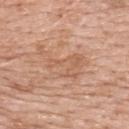biopsy status: catalogued during a skin exam; not biopsied | illumination: white-light illumination | image: 15 mm crop, total-body photography | TBP lesion metrics: a shape eccentricity near 0.85 and a symmetry-axis asymmetry near 0.45; an average lesion color of about L≈60 a*≈21 b*≈32 (CIELAB) and roughly 7 lightness units darker than nearby skin | body site: the upper back | patient: female, in their 60s.